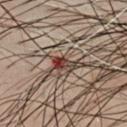anatomic site: the chest | image: 15 mm crop, total-body photography | lighting: cross-polarized | automated metrics: about 15 CIELAB-L* units darker than the surrounding skin; a classifier nevus-likeness of about 75/100 and a lesion-detection confidence of about 100/100 | subject: male, about 50 years old.